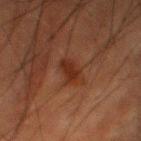biopsy status: catalogued during a skin exam; not biopsied | image: ~15 mm crop, total-body skin-cancer survey | tile lighting: cross-polarized | subject: male, aged approximately 50 | TBP lesion metrics: a lesion area of about 5.5 mm², a shape eccentricity near 0.65, and two-axis asymmetry of about 0.25; a color-variation rating of about 1.5/10 and a peripheral color-asymmetry measure near 0.5; a classifier nevus-likeness of about 15/100 and a lesion-detection confidence of about 100/100 | body site: the left lower leg | lesion diameter: ~3 mm (longest diameter).